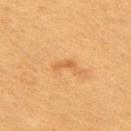Impression:
No biopsy was performed on this lesion — it was imaged during a full skin examination and was not determined to be concerning.
Clinical summary:
A 15 mm close-up extracted from a 3D total-body photography capture. Imaged with cross-polarized lighting. The total-body-photography lesion software estimated a border-irregularity rating of about 4/10, a color-variation rating of about 0/10, and peripheral color asymmetry of about 0. The software also gave an automated nevus-likeness rating near 5 out of 100 and a lesion-detection confidence of about 100/100. The lesion is located on the right upper arm. The subject is a female aged approximately 40. Longest diameter approximately 2.5 mm.